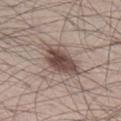notes = total-body-photography surveillance lesion; no biopsy | image source = ~15 mm crop, total-body skin-cancer survey | subject = male, approximately 30 years of age | site = the right lower leg | illumination = white-light illumination.